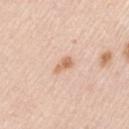workup: catalogued during a skin exam; not biopsied | body site: the left upper arm | imaging modality: ~15 mm crop, total-body skin-cancer survey | patient: male, in their mid- to late 40s.